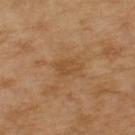The lesion was tiled from a total-body skin photograph and was not biopsied.
The recorded lesion diameter is about 3 mm.
A male subject, in their mid- to late 60s.
Imaged with cross-polarized lighting.
A 15 mm close-up tile from a total-body photography series done for melanoma screening.